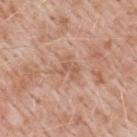Captured during whole-body skin photography for melanoma surveillance; the lesion was not biopsied. Captured under white-light illumination. From the upper back. A close-up tile cropped from a whole-body skin photograph, about 15 mm across. Approximately 3 mm at its widest. A male patient, aged 48 to 52. Automated tile analysis of the lesion measured an average lesion color of about L≈58 a*≈21 b*≈31 (CIELAB), about 7 CIELAB-L* units darker than the surrounding skin, and a normalized lesion–skin contrast near 5. The software also gave a border-irregularity rating of about 6/10. It also reported a nevus-likeness score of about 0/100.